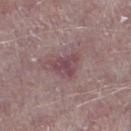notes: imaged on a skin check; not biopsied